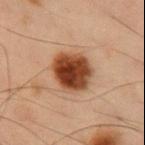The lesion was tiled from a total-body skin photograph and was not biopsied. The lesion is on the chest. The subject is a male roughly 55 years of age. The lesion-visualizer software estimated an average lesion color of about L≈37 a*≈19 b*≈28 (CIELAB), roughly 15 lightness units darker than nearby skin, and a lesion-to-skin contrast of about 12.5 (normalized; higher = more distinct). Approximately 5 mm at its widest. A lesion tile, about 15 mm wide, cut from a 3D total-body photograph. Captured under cross-polarized illumination.